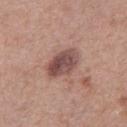Notes:
• location · the right thigh
• subject · female, aged 38–42
• TBP lesion metrics · a footprint of about 10 mm², a shape eccentricity near 0.75, and a symmetry-axis asymmetry near 0.15; a mean CIELAB color near L≈48 a*≈21 b*≈22 and a lesion–skin lightness drop of about 13; a nevus-likeness score of about 30/100 and lesion-presence confidence of about 100/100
• tile lighting · white-light illumination
• image source · total-body-photography crop, ~15 mm field of view